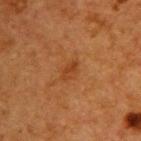notes = catalogued during a skin exam; not biopsied | subject = female, aged approximately 40 | automated metrics = an average lesion color of about L≈36 a*≈24 b*≈36 (CIELAB), roughly 6 lightness units darker than nearby skin, and a normalized lesion–skin contrast near 6; a classifier nevus-likeness of about 20/100 | lesion diameter = ≈2.5 mm | tile lighting = cross-polarized illumination | body site = the back | acquisition = 15 mm crop, total-body photography.